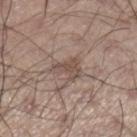Impression:
Recorded during total-body skin imaging; not selected for excision or biopsy.
Image and clinical context:
The patient is a male about 55 years old. The lesion is located on the right lower leg. Automated tile analysis of the lesion measured an outline eccentricity of about 0.8 (0 = round, 1 = elongated). The software also gave a lesion color around L≈50 a*≈15 b*≈22 in CIELAB and roughly 8 lightness units darker than nearby skin. A 15 mm crop from a total-body photograph taken for skin-cancer surveillance.